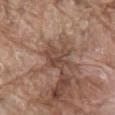{"biopsy_status": "not biopsied; imaged during a skin examination", "site": "mid back", "lesion_size": {"long_diameter_mm_approx": 3.5}, "image": {"source": "total-body photography crop", "field_of_view_mm": 15}, "automated_metrics": {"area_mm2_approx": 7.5, "eccentricity": 0.6, "shape_asymmetry": 0.3, "color_variation_0_10": 4.0}, "patient": {"sex": "male", "age_approx": 80}}